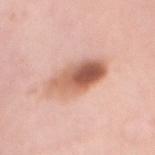No biopsy was performed on this lesion — it was imaged during a full skin examination and was not determined to be concerning. Located on the chest. The tile uses white-light illumination. The total-body-photography lesion software estimated a mean CIELAB color near L≈61 a*≈23 b*≈31, about 15 CIELAB-L* units darker than the surrounding skin, and a normalized border contrast of about 9.5. And it measured a color-variation rating of about 10/10 and radial color variation of about 5. The software also gave a classifier nevus-likeness of about 95/100 and lesion-presence confidence of about 100/100. Approximately 6.5 mm at its widest. A 15 mm crop from a total-body photograph taken for skin-cancer surveillance. The subject is a female aged 48 to 52.